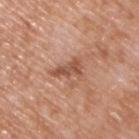{"biopsy_status": "not biopsied; imaged during a skin examination", "patient": {"sex": "male", "age_approx": 50}, "image": {"source": "total-body photography crop", "field_of_view_mm": 15}, "lighting": "white-light", "site": "upper back", "lesion_size": {"long_diameter_mm_approx": 4.0}}